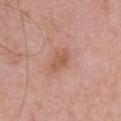The total-body-photography lesion software estimated a lesion area of about 4.5 mm², an outline eccentricity of about 0.8 (0 = round, 1 = elongated), and a shape-asymmetry score of about 0.2 (0 = symmetric). The analysis additionally found border irregularity of about 2.5 on a 0–10 scale, a within-lesion color-variation index near 2/10, and radial color variation of about 1. Approximately 3 mm at its widest. This image is a 15 mm lesion crop taken from a total-body photograph. The lesion is on the upper back. A female patient, aged 28 to 32. This is a white-light tile.